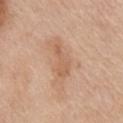Captured during whole-body skin photography for melanoma surveillance; the lesion was not biopsied. The recorded lesion diameter is about 5 mm. A 15 mm crop from a total-body photograph taken for skin-cancer surveillance. The tile uses white-light illumination. The lesion is on the chest. The patient is a female aged 63 to 67.